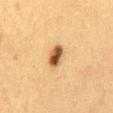Part of a total-body skin-imaging series; this lesion was reviewed on a skin check and was not flagged for biopsy.
A 15 mm close-up extracted from a 3D total-body photography capture.
A female subject, in their 40s.
Automated image analysis of the tile measured a footprint of about 5 mm², a shape eccentricity near 0.8, and a shape-asymmetry score of about 0.2 (0 = symmetric). The analysis additionally found a lesion color around L≈46 a*≈19 b*≈35 in CIELAB, a lesion–skin lightness drop of about 17, and a lesion-to-skin contrast of about 12.5 (normalized; higher = more distinct). The software also gave a nevus-likeness score of about 100/100.
Measured at roughly 3 mm in maximum diameter.
On the mid back.
The tile uses cross-polarized illumination.